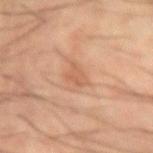The lesion's longest dimension is about 3 mm.
The total-body-photography lesion software estimated an area of roughly 4 mm², a shape eccentricity near 0.75, and a shape-asymmetry score of about 0.35 (0 = symmetric). It also reported a border-irregularity index near 3.5/10, a color-variation rating of about 1.5/10, and peripheral color asymmetry of about 0.5.
The tile uses cross-polarized illumination.
On the right forearm.
A male patient aged 58–62.
Cropped from a total-body skin-imaging series; the visible field is about 15 mm.